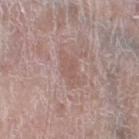Image and clinical context: A 15 mm close-up tile from a total-body photography series done for melanoma screening. This is a white-light tile. The lesion's longest dimension is about 4 mm. The lesion is located on the leg. A male subject about 80 years old.